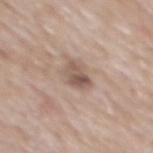{
  "biopsy_status": "not biopsied; imaged during a skin examination",
  "lighting": "white-light",
  "patient": {
    "sex": "male",
    "age_approx": 65
  },
  "image": {
    "source": "total-body photography crop",
    "field_of_view_mm": 15
  },
  "lesion_size": {
    "long_diameter_mm_approx": 3.0
  },
  "site": "back",
  "automated_metrics": {
    "eccentricity": 0.7,
    "shape_asymmetry": 0.25,
    "cielab_L": 54,
    "cielab_a": 16,
    "cielab_b": 24,
    "vs_skin_darker_L": 11.0,
    "vs_skin_contrast_norm": 7.5,
    "border_irregularity_0_10": 2.5,
    "color_variation_0_10": 6.5,
    "peripheral_color_asymmetry": 2.5,
    "lesion_detection_confidence_0_100": 100
  }
}